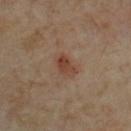| key | value |
|---|---|
| biopsy status | total-body-photography surveillance lesion; no biopsy |
| image source | ~15 mm tile from a whole-body skin photo |
| lesion diameter | ≈3 mm |
| automated lesion analysis | a lesion area of about 5 mm² and a symmetry-axis asymmetry near 0.25 |
| patient | male, in their 70s |
| lighting | cross-polarized illumination |
| location | the chest |